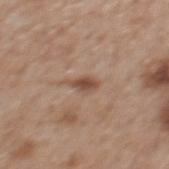• notes · total-body-photography surveillance lesion; no biopsy
• patient · male, in their mid-60s
• lighting · white-light
• anatomic site · the back
• image source · total-body-photography crop, ~15 mm field of view
• diameter · ~3 mm (longest diameter)